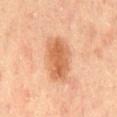A male patient, aged 43–47. Automated tile analysis of the lesion measured roughly 11 lightness units darker than nearby skin. The analysis additionally found an automated nevus-likeness rating near 90 out of 100 and a detector confidence of about 100 out of 100 that the crop contains a lesion. On the mid back. Captured under cross-polarized illumination. A roughly 15 mm field-of-view crop from a total-body skin photograph. Approximately 5 mm at its widest.